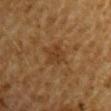Assessment:
Recorded during total-body skin imaging; not selected for excision or biopsy.
Acquisition and patient details:
Approximately 2.5 mm at its widest. A male subject, aged 83 or over. Imaged with cross-polarized lighting. A region of skin cropped from a whole-body photographic capture, roughly 15 mm wide. Located on the right upper arm.